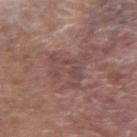The lesion was photographed on a routine skin check and not biopsied; there is no pathology result. The subject is a male aged 63–67. Measured at roughly 4.5 mm in maximum diameter. The lesion is on the left forearm. A 15 mm close-up tile from a total-body photography series done for melanoma screening. This is a white-light tile.